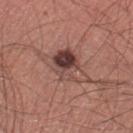Q: Was this lesion biopsied?
A: no biopsy performed (imaged during a skin exam)
Q: How was this image acquired?
A: ~15 mm tile from a whole-body skin photo
Q: What lighting was used for the tile?
A: white-light illumination
Q: Lesion size?
A: ≈5.5 mm
Q: What is the anatomic site?
A: the right thigh
Q: What are the patient's age and sex?
A: male, about 45 years old